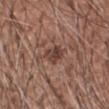{
  "biopsy_status": "not biopsied; imaged during a skin examination",
  "site": "right forearm",
  "automated_metrics": {
    "lesion_detection_confidence_0_100": 100
  },
  "image": {
    "source": "total-body photography crop",
    "field_of_view_mm": 15
  },
  "lesion_size": {
    "long_diameter_mm_approx": 3.0
  },
  "lighting": "white-light",
  "patient": {
    "sex": "male",
    "age_approx": 60
  }
}